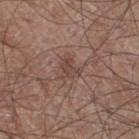Imaged during a routine full-body skin examination; the lesion was not biopsied and no histopathology is available. A male subject aged around 60. The lesion-visualizer software estimated a footprint of about 4.5 mm², an eccentricity of roughly 0.4, and a symmetry-axis asymmetry near 0.5. And it measured a classifier nevus-likeness of about 0/100 and lesion-presence confidence of about 100/100. About 2.5 mm across. A roughly 15 mm field-of-view crop from a total-body skin photograph. Located on the right thigh.